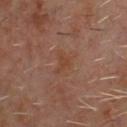Clinical impression:
Captured during whole-body skin photography for melanoma surveillance; the lesion was not biopsied.
Clinical summary:
Imaged with cross-polarized lighting. A 15 mm crop from a total-body photograph taken for skin-cancer surveillance. The lesion is on the chest. Measured at roughly 2.5 mm in maximum diameter. A male patient, aged approximately 60.